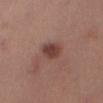Clinical impression: The lesion was photographed on a routine skin check and not biopsied; there is no pathology result. Context: The lesion is located on the right thigh. Longest diameter approximately 3.5 mm. A roughly 15 mm field-of-view crop from a total-body skin photograph. The subject is a female aged 38–42.